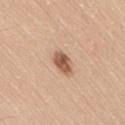Q: Is there a histopathology result?
A: no biopsy performed (imaged during a skin exam)
Q: Lesion location?
A: the lower back
Q: What did automated image analysis measure?
A: a normalized lesion–skin contrast near 9.5
Q: Illumination type?
A: white-light
Q: What are the patient's age and sex?
A: male, aged approximately 40
Q: What is the lesion's diameter?
A: about 3 mm
Q: What kind of image is this?
A: 15 mm crop, total-body photography